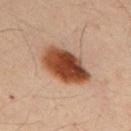biopsy status: total-body-photography surveillance lesion; no biopsy.